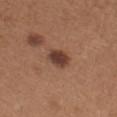Part of a total-body skin-imaging series; this lesion was reviewed on a skin check and was not flagged for biopsy.
The subject is a male aged around 65.
Measured at roughly 2.5 mm in maximum diameter.
Imaged with white-light lighting.
A roughly 15 mm field-of-view crop from a total-body skin photograph.
Automated tile analysis of the lesion measured a lesion color around L≈39 a*≈20 b*≈26 in CIELAB and a normalized lesion–skin contrast near 10.5. It also reported a classifier nevus-likeness of about 75/100 and lesion-presence confidence of about 100/100.
On the left forearm.